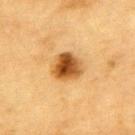{"site": "upper back", "image": {"source": "total-body photography crop", "field_of_view_mm": 15}, "lesion_size": {"long_diameter_mm_approx": 3.5}, "patient": {"sex": "male", "age_approx": "85+"}, "lighting": "cross-polarized", "automated_metrics": {"area_mm2_approx": 9.5, "eccentricity": 0.5, "shape_asymmetry": 0.2, "border_irregularity_0_10": 2.0, "color_variation_0_10": 7.0, "peripheral_color_asymmetry": 2.0, "nevus_likeness_0_100": 100}}